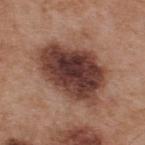Imaged during a routine full-body skin examination; the lesion was not biopsied and no histopathology is available.
Captured under white-light illumination.
Cropped from a whole-body photographic skin survey; the tile spans about 15 mm.
A male subject, roughly 55 years of age.
From the upper back.